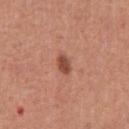Q: Was a biopsy performed?
A: imaged on a skin check; not biopsied
Q: What did automated image analysis measure?
A: a footprint of about 3.5 mm², an outline eccentricity of about 0.8 (0 = round, 1 = elongated), and two-axis asymmetry of about 0.2; an average lesion color of about L≈48 a*≈26 b*≈30 (CIELAB), a lesion–skin lightness drop of about 13, and a normalized border contrast of about 9; border irregularity of about 1.5 on a 0–10 scale, a within-lesion color-variation index near 1.5/10, and a peripheral color-asymmetry measure near 0.5
Q: How was the tile lit?
A: white-light illumination
Q: Who is the patient?
A: male, aged approximately 65
Q: Where on the body is the lesion?
A: the chest
Q: What is the lesion's diameter?
A: ≈2.5 mm
Q: What is the imaging modality?
A: ~15 mm tile from a whole-body skin photo